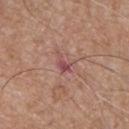| field | value |
|---|---|
| biopsy status | total-body-photography surveillance lesion; no biopsy |
| site | the front of the torso |
| image source | 15 mm crop, total-body photography |
| patient | male, approximately 55 years of age |